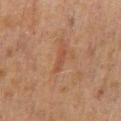notes: catalogued during a skin exam; not biopsied